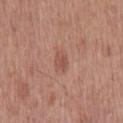  lighting: white-light
  image:
    source: total-body photography crop
    field_of_view_mm: 15
  patient:
    sex: male
    age_approx: 60
  automated_metrics:
    area_mm2_approx: 3.5
    eccentricity: 0.8
    shape_asymmetry: 0.2
    cielab_L: 52
    cielab_a: 24
    cielab_b: 27
    border_irregularity_0_10: 2.0
    color_variation_0_10: 2.5
    peripheral_color_asymmetry: 1.0
    nevus_likeness_0_100: 5
    lesion_detection_confidence_0_100: 100
  site: mid back
  lesion_size:
    long_diameter_mm_approx: 2.5